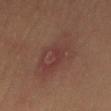* biopsy status: imaged on a skin check; not biopsied
* image: ~15 mm crop, total-body skin-cancer survey
* automated metrics: an area of roughly 21 mm², an outline eccentricity of about 0.9 (0 = round, 1 = elongated), and a symmetry-axis asymmetry near 0.2; an average lesion color of about L≈38 a*≈20 b*≈22 (CIELAB), roughly 6 lightness units darker than nearby skin, and a normalized border contrast of about 6; a color-variation rating of about 6/10
* body site: the left thigh
* subject: male, in their 50s
* lighting: cross-polarized